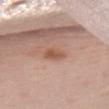The lesion was photographed on a routine skin check and not biopsied; there is no pathology result.
The tile uses white-light illumination.
Automated image analysis of the tile measured a lesion area of about 4 mm² and a shape eccentricity near 0.85. It also reported a mean CIELAB color near L≈55 a*≈22 b*≈30, roughly 8 lightness units darker than nearby skin, and a lesion-to-skin contrast of about 7 (normalized; higher = more distinct).
A female subject aged 63 to 67.
About 3 mm across.
Cropped from a whole-body photographic skin survey; the tile spans about 15 mm.
The lesion is located on the chest.